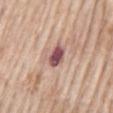notes = catalogued during a skin exam; not biopsied
diameter = ~3 mm (longest diameter)
patient = male, aged 63–67
image = 15 mm crop, total-body photography
location = the back
illumination = white-light